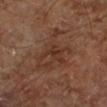notes=total-body-photography surveillance lesion; no biopsy
acquisition=~15 mm crop, total-body skin-cancer survey
lesion diameter=~7.5 mm (longest diameter)
illumination=cross-polarized illumination
location=the left lower leg
patient=aged around 65